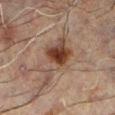biopsy_status: not biopsied; imaged during a skin examination
image:
  source: total-body photography crop
  field_of_view_mm: 15
site: leg
automated_metrics:
  area_mm2_approx: 15.0
  shape_asymmetry: 0.4
  cielab_L: 32
  cielab_a: 14
  cielab_b: 21
  vs_skin_darker_L: 9.0
  vs_skin_contrast_norm: 9.0
  color_variation_0_10: 6.0
  peripheral_color_asymmetry: 1.5
  lesion_detection_confidence_0_100: 100
lighting: cross-polarized
patient:
  sex: male
  age_approx: 60